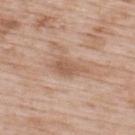This lesion was catalogued during total-body skin photography and was not selected for biopsy. Captured under white-light illumination. The recorded lesion diameter is about 4.5 mm. The total-body-photography lesion software estimated an area of roughly 6.5 mm², an outline eccentricity of about 0.9 (0 = round, 1 = elongated), and a symmetry-axis asymmetry near 0.35. It also reported a color-variation rating of about 2/10 and a peripheral color-asymmetry measure near 0.5. It also reported a detector confidence of about 100 out of 100 that the crop contains a lesion. Cropped from a whole-body photographic skin survey; the tile spans about 15 mm. Located on the upper back. A male subject, in their 50s.